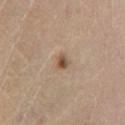– biopsy status · total-body-photography surveillance lesion; no biopsy
– imaging modality · 15 mm crop, total-body photography
– diameter · ~2 mm (longest diameter)
– patient · male, aged 53 to 57
– site · the left lower leg